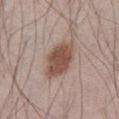Impression: Captured during whole-body skin photography for melanoma surveillance; the lesion was not biopsied. Clinical summary: This is a white-light tile. A male subject, aged 68 to 72. A region of skin cropped from a whole-body photographic capture, roughly 15 mm wide. From the abdomen. The lesion's longest dimension is about 4.5 mm. Automated image analysis of the tile measured roughly 13 lightness units darker than nearby skin and a normalized lesion–skin contrast near 9.5. The software also gave border irregularity of about 2.5 on a 0–10 scale, a color-variation rating of about 3/10, and peripheral color asymmetry of about 1. And it measured a classifier nevus-likeness of about 100/100.